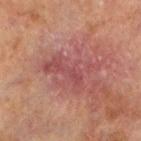Clinical impression: Captured during whole-body skin photography for melanoma surveillance; the lesion was not biopsied. Acquisition and patient details: Automated image analysis of the tile measured a lesion color around L≈39 a*≈23 b*≈19 in CIELAB. The software also gave border irregularity of about 8.5 on a 0–10 scale, internal color variation of about 2.5 on a 0–10 scale, and a peripheral color-asymmetry measure near 1. The tile uses cross-polarized illumination. Longest diameter approximately 6.5 mm. On the leg. A male patient, about 70 years old. A 15 mm close-up tile from a total-body photography series done for melanoma screening.